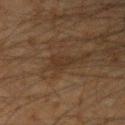The lesion was tiled from a total-body skin photograph and was not biopsied.